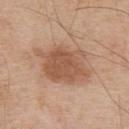This lesion was catalogued during total-body skin photography and was not selected for biopsy.
Automated image analysis of the tile measured an area of roughly 22 mm². The analysis additionally found a lesion color around L≈55 a*≈21 b*≈32 in CIELAB, about 11 CIELAB-L* units darker than the surrounding skin, and a lesion-to-skin contrast of about 7.5 (normalized; higher = more distinct). The analysis additionally found a classifier nevus-likeness of about 70/100.
The subject is a male about 55 years old.
Cropped from a whole-body photographic skin survey; the tile spans about 15 mm.
This is a white-light tile.
On the upper back.